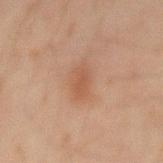- notes · imaged on a skin check; not biopsied
- tile lighting · cross-polarized illumination
- image-analysis metrics · a mean CIELAB color near L≈44 a*≈18 b*≈27, about 6 CIELAB-L* units darker than the surrounding skin, and a lesion-to-skin contrast of about 5.5 (normalized; higher = more distinct); a border-irregularity rating of about 2.5/10; a classifier nevus-likeness of about 45/100 and lesion-presence confidence of about 100/100
- body site · the back
- acquisition · ~15 mm crop, total-body skin-cancer survey
- patient · male, in their mid- to late 40s